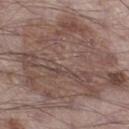tile lighting: white-light | image-analysis metrics: border irregularity of about 7.5 on a 0–10 scale and a peripheral color-asymmetry measure near 2.5; a nevus-likeness score of about 0/100 and a lesion-detection confidence of about 90/100 | diameter: ~14.5 mm (longest diameter) | subject: male, in their 70s | imaging modality: total-body-photography crop, ~15 mm field of view | body site: the left thigh.